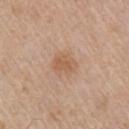{
  "biopsy_status": "not biopsied; imaged during a skin examination",
  "image": {
    "source": "total-body photography crop",
    "field_of_view_mm": 15
  },
  "lesion_size": {
    "long_diameter_mm_approx": 2.5
  },
  "lighting": "white-light",
  "patient": {
    "sex": "male",
    "age_approx": 55
  },
  "site": "arm",
  "automated_metrics": {
    "area_mm2_approx": 4.5,
    "eccentricity": 0.7,
    "shape_asymmetry": 0.3,
    "border_irregularity_0_10": 2.5,
    "color_variation_0_10": 2.0,
    "peripheral_color_asymmetry": 1.0,
    "nevus_likeness_0_100": 15,
    "lesion_detection_confidence_0_100": 100
  }
}